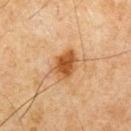follow-up: imaged on a skin check; not biopsied | image: ~15 mm tile from a whole-body skin photo | size: about 4 mm | subject: male, approximately 65 years of age | body site: the front of the torso | illumination: cross-polarized illumination | automated lesion analysis: a lesion area of about 7.5 mm², a shape eccentricity near 0.7, and a symmetry-axis asymmetry near 0.15; a lesion-to-skin contrast of about 10 (normalized; higher = more distinct); a border-irregularity rating of about 1.5/10 and a peripheral color-asymmetry measure near 1; a detector confidence of about 100 out of 100 that the crop contains a lesion.